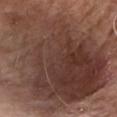Q: Was this lesion biopsied?
A: catalogued during a skin exam; not biopsied
Q: How was this image acquired?
A: ~15 mm tile from a whole-body skin photo
Q: Who is the patient?
A: male, aged 78 to 82
Q: Where on the body is the lesion?
A: the chest